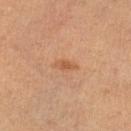| feature | finding |
|---|---|
| biopsy status | total-body-photography surveillance lesion; no biopsy |
| acquisition | ~15 mm tile from a whole-body skin photo |
| subject | female, aged approximately 60 |
| tile lighting | cross-polarized illumination |
| automated metrics | a footprint of about 3 mm², an eccentricity of roughly 0.9, and a symmetry-axis asymmetry near 0.2; a classifier nevus-likeness of about 15/100 and a detector confidence of about 100 out of 100 that the crop contains a lesion |
| site | the left lower leg |
| lesion size | ~3 mm (longest diameter) |